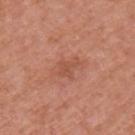biopsy status=no biopsy performed (imaged during a skin exam)
illumination=white-light
body site=the left upper arm
diameter=about 3 mm
patient=male, approximately 70 years of age
acquisition=~15 mm crop, total-body skin-cancer survey